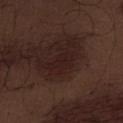Assessment: Imaged during a routine full-body skin examination; the lesion was not biopsied and no histopathology is available. Background: The recorded lesion diameter is about 3.5 mm. A 15 mm close-up tile from a total-body photography series done for melanoma screening. The patient is a male in their 70s. On the abdomen. The tile uses white-light illumination.